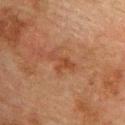Assessment:
The lesion was photographed on a routine skin check and not biopsied; there is no pathology result.
Background:
The tile uses cross-polarized illumination. An algorithmic analysis of the crop reported a footprint of about 4 mm² and a shape eccentricity near 0.85. Longest diameter approximately 3.5 mm. On the chest. Cropped from a whole-body photographic skin survey; the tile spans about 15 mm. A male subject, aged approximately 75.